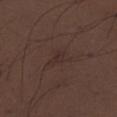{
  "biopsy_status": "not biopsied; imaged during a skin examination",
  "image": {
    "source": "total-body photography crop",
    "field_of_view_mm": 15
  },
  "patient": {
    "sex": "male",
    "age_approx": 30
  },
  "site": "left lower leg"
}